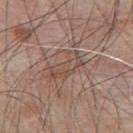Q: Was this lesion biopsied?
A: catalogued during a skin exam; not biopsied
Q: Lesion size?
A: ~4.5 mm (longest diameter)
Q: Who is the patient?
A: male, aged 48 to 52
Q: What is the anatomic site?
A: the upper back
Q: What kind of image is this?
A: 15 mm crop, total-body photography